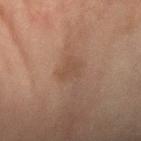biopsy status = no biopsy performed (imaged during a skin exam)
diameter = ~2.5 mm (longest diameter)
subject = female, aged 58 to 62
lighting = cross-polarized
anatomic site = the left forearm
acquisition = 15 mm crop, total-body photography
automated metrics = a mean CIELAB color near L≈42 a*≈16 b*≈25; a border-irregularity index near 3.5/10 and a peripheral color-asymmetry measure near 0.5